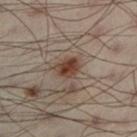Imaged during a routine full-body skin examination; the lesion was not biopsied and no histopathology is available. Cropped from a total-body skin-imaging series; the visible field is about 15 mm. The subject is a male aged around 50. From the leg.